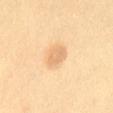Recorded during total-body skin imaging; not selected for excision or biopsy.